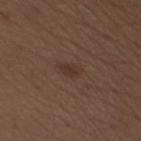biopsy status: imaged on a skin check; not biopsied | subject: male, aged around 70 | imaging modality: total-body-photography crop, ~15 mm field of view | site: the right upper arm | lesion size: ~2.5 mm (longest diameter) | illumination: white-light.